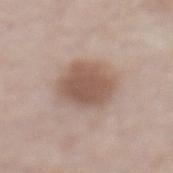This is a white-light tile.
A 15 mm close-up tile from a total-body photography series done for melanoma screening.
The lesion is located on the abdomen.
Automated image analysis of the tile measured a lesion color around L≈54 a*≈17 b*≈25 in CIELAB, a lesion–skin lightness drop of about 12, and a normalized lesion–skin contrast near 8.
The subject is a male about 55 years old.
About 5 mm across.